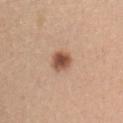Q: Is there a histopathology result?
A: total-body-photography surveillance lesion; no biopsy
Q: What is the imaging modality?
A: ~15 mm crop, total-body skin-cancer survey
Q: Who is the patient?
A: female, aged around 30
Q: Where on the body is the lesion?
A: the chest
Q: Automated lesion metrics?
A: a lesion area of about 6 mm² and an eccentricity of roughly 0.4; a lesion color around L≈53 a*≈21 b*≈31 in CIELAB, roughly 15 lightness units darker than nearby skin, and a normalized border contrast of about 10; internal color variation of about 4.5 on a 0–10 scale and radial color variation of about 1
Q: What lighting was used for the tile?
A: white-light illumination
Q: What is the lesion's diameter?
A: ~2.5 mm (longest diameter)